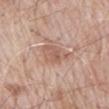The lesion was tiled from a total-body skin photograph and was not biopsied.